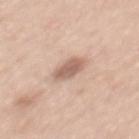Recorded during total-body skin imaging; not selected for excision or biopsy.
A 15 mm close-up tile from a total-body photography series done for melanoma screening.
From the mid back.
Approximately 4 mm at its widest.
Captured under white-light illumination.
A male subject, aged around 50.